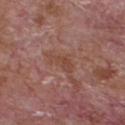Notes:
• notes — catalogued during a skin exam; not biopsied